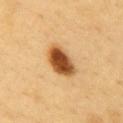Imaged during a routine full-body skin examination; the lesion was not biopsied and no histopathology is available. This is a cross-polarized tile. A 15 mm close-up extracted from a 3D total-body photography capture. The subject is a male aged approximately 60. Measured at roughly 4.5 mm in maximum diameter. On the chest.